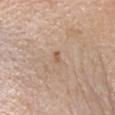Recorded during total-body skin imaging; not selected for excision or biopsy.